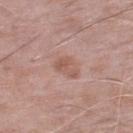• lesion size · about 3 mm
• site · the left lower leg
• lighting · white-light illumination
• image · total-body-photography crop, ~15 mm field of view
• automated metrics · a lesion area of about 6 mm², an outline eccentricity of about 0.75 (0 = round, 1 = elongated), and two-axis asymmetry of about 0.2; a border-irregularity index near 2/10, a color-variation rating of about 3/10, and a peripheral color-asymmetry measure near 1
• patient · male, aged 73 to 77